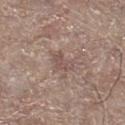notes: catalogued during a skin exam; not biopsied | TBP lesion metrics: a symmetry-axis asymmetry near 0.45; a border-irregularity index near 4.5/10 and a color-variation rating of about 1.5/10; lesion-presence confidence of about 95/100 | imaging modality: total-body-photography crop, ~15 mm field of view | lighting: white-light | subject: male, aged around 65 | size: ~3 mm (longest diameter) | body site: the right lower leg.